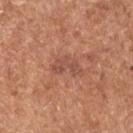Located on the upper back. A male subject aged approximately 65. A 15 mm close-up tile from a total-body photography series done for melanoma screening.